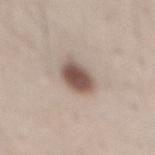Part of a total-body skin-imaging series; this lesion was reviewed on a skin check and was not flagged for biopsy. A 15 mm crop from a total-body photograph taken for skin-cancer surveillance. The lesion-visualizer software estimated a shape eccentricity near 0.45 and a symmetry-axis asymmetry near 0.15. And it measured a border-irregularity rating of about 1.5/10 and peripheral color asymmetry of about 1. It also reported a nevus-likeness score of about 100/100 and a detector confidence of about 100 out of 100 that the crop contains a lesion. From the lower back. The subject is a male in their mid- to late 30s.